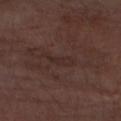Assessment: Imaged during a routine full-body skin examination; the lesion was not biopsied and no histopathology is available. Background: A male subject, in their mid-70s. On the right forearm. This image is a 15 mm lesion crop taken from a total-body photograph. Longest diameter approximately 3.5 mm. Captured under white-light illumination. Automated image analysis of the tile measured an outline eccentricity of about 0.95 (0 = round, 1 = elongated). And it measured a border-irregularity index near 5/10 and peripheral color asymmetry of about 0.